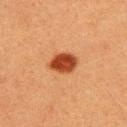biopsy status: no biopsy performed (imaged during a skin exam) | size: about 3.5 mm | anatomic site: the upper back | automated lesion analysis: a lesion area of about 7.5 mm², a shape eccentricity near 0.65, and two-axis asymmetry of about 0.2; a lesion–skin lightness drop of about 15 and a normalized lesion–skin contrast near 12; border irregularity of about 1.5 on a 0–10 scale, a within-lesion color-variation index near 3/10, and a peripheral color-asymmetry measure near 1; a classifier nevus-likeness of about 100/100 | illumination: cross-polarized | imaging modality: ~15 mm crop, total-body skin-cancer survey | subject: male, in their 40s.